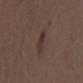This lesion was catalogued during total-body skin photography and was not selected for biopsy.
A close-up tile cropped from a whole-body skin photograph, about 15 mm across.
Automated tile analysis of the lesion measured a lesion area of about 3.5 mm², a shape eccentricity near 0.85, and a symmetry-axis asymmetry near 0.35. The software also gave a border-irregularity index near 3.5/10 and peripheral color asymmetry of about 0.5.
This is a white-light tile.
The lesion is on the right upper arm.
The lesion's longest dimension is about 3 mm.
A male subject aged approximately 70.